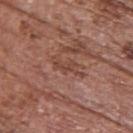Captured during whole-body skin photography for melanoma surveillance; the lesion was not biopsied.
Captured under white-light illumination.
About 4.5 mm across.
Automated image analysis of the tile measured a footprint of about 5 mm², a shape eccentricity near 0.9, and a symmetry-axis asymmetry near 0.5. The software also gave roughly 7 lightness units darker than nearby skin and a normalized lesion–skin contrast near 6. And it measured border irregularity of about 6.5 on a 0–10 scale, internal color variation of about 1.5 on a 0–10 scale, and a peripheral color-asymmetry measure near 0.5.
A 15 mm close-up tile from a total-body photography series done for melanoma screening.
On the upper back.
The subject is a female roughly 65 years of age.